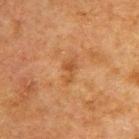workup: imaged on a skin check; not biopsied | illumination: cross-polarized | diameter: about 3 mm | acquisition: ~15 mm tile from a whole-body skin photo | patient: male, aged 48 to 52 | body site: the upper back | automated lesion analysis: a border-irregularity rating of about 4.5/10, a color-variation rating of about 1/10, and peripheral color asymmetry of about 0.